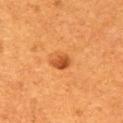Assessment:
Recorded during total-body skin imaging; not selected for excision or biopsy.
Acquisition and patient details:
A lesion tile, about 15 mm wide, cut from a 3D total-body photograph. The lesion is located on the right upper arm. A female subject aged 53 to 57.